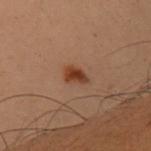Q: Was this lesion biopsied?
A: imaged on a skin check; not biopsied
Q: Where on the body is the lesion?
A: the right upper arm
Q: What is the lesion's diameter?
A: ~2.5 mm (longest diameter)
Q: Patient demographics?
A: female, approximately 50 years of age
Q: How was this image acquired?
A: ~15 mm crop, total-body skin-cancer survey